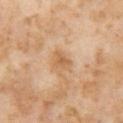Assessment:
This lesion was catalogued during total-body skin photography and was not selected for biopsy.
Image and clinical context:
The lesion is on the left thigh. A region of skin cropped from a whole-body photographic capture, roughly 15 mm wide. Longest diameter approximately 2.5 mm. A female subject in their mid-50s. The tile uses cross-polarized illumination. The total-body-photography lesion software estimated peripheral color asymmetry of about 1.